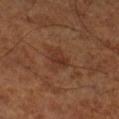Captured during whole-body skin photography for melanoma surveillance; the lesion was not biopsied. Measured at roughly 2.5 mm in maximum diameter. Captured under cross-polarized illumination. A roughly 15 mm field-of-view crop from a total-body skin photograph. A subject roughly 65 years of age. Located on the leg. Automated image analysis of the tile measured an automated nevus-likeness rating near 10 out of 100 and a detector confidence of about 100 out of 100 that the crop contains a lesion.